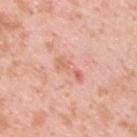The lesion was photographed on a routine skin check and not biopsied; there is no pathology result.
The lesion is on the upper back.
Measured at roughly 4.5 mm in maximum diameter.
A lesion tile, about 15 mm wide, cut from a 3D total-body photograph.
A male subject, aged approximately 35.
Captured under white-light illumination.